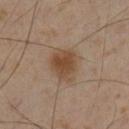follow-up = total-body-photography surveillance lesion; no biopsy
patient = male, about 55 years old
automated lesion analysis = a mean CIELAB color near L≈46 a*≈17 b*≈30, a lesion–skin lightness drop of about 9, and a normalized lesion–skin contrast near 8; peripheral color asymmetry of about 1.5; a nevus-likeness score of about 95/100 and lesion-presence confidence of about 100/100
lighting = cross-polarized
image = ~15 mm crop, total-body skin-cancer survey
body site = the left lower leg
diameter = about 4 mm